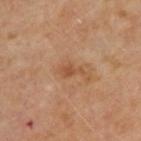notes: catalogued during a skin exam; not biopsied
acquisition: ~15 mm tile from a whole-body skin photo
subject: male, aged around 65
automated metrics: a nevus-likeness score of about 0/100 and lesion-presence confidence of about 100/100
body site: the upper back
diameter: ≈2.5 mm